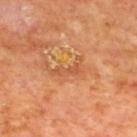The lesion is on the upper back. A male patient aged 68 to 72. A 15 mm close-up tile from a total-body photography series done for melanoma screening.